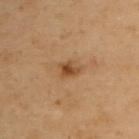Imaged during a routine full-body skin examination; the lesion was not biopsied and no histopathology is available. About 2.5 mm across. A lesion tile, about 15 mm wide, cut from a 3D total-body photograph. The lesion is on the upper back. A female subject, aged 58–62. The tile uses cross-polarized illumination.